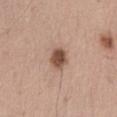Q: Was this lesion biopsied?
A: total-body-photography surveillance lesion; no biopsy
Q: Who is the patient?
A: male, roughly 55 years of age
Q: What did automated image analysis measure?
A: a shape eccentricity near 0.4 and a symmetry-axis asymmetry near 0.2; an automated nevus-likeness rating near 95 out of 100 and a lesion-detection confidence of about 100/100
Q: What is the lesion's diameter?
A: ≈2.5 mm
Q: Where on the body is the lesion?
A: the abdomen
Q: How was this image acquired?
A: 15 mm crop, total-body photography
Q: Illumination type?
A: white-light